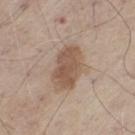The lesion was tiled from a total-body skin photograph and was not biopsied. The lesion is on the left thigh. Longest diameter approximately 5.5 mm. A 15 mm close-up extracted from a 3D total-body photography capture. Imaged with white-light lighting. A male subject, approximately 60 years of age.